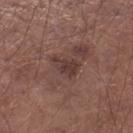This lesion was catalogued during total-body skin photography and was not selected for biopsy. A close-up tile cropped from a whole-body skin photograph, about 15 mm across. Located on the right lower leg. A male patient aged 53–57.